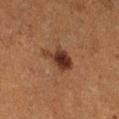Image and clinical context: Measured at roughly 3.5 mm in maximum diameter. A female subject, in their 50s. The lesion is on the leg. The tile uses cross-polarized illumination. A region of skin cropped from a whole-body photographic capture, roughly 15 mm wide.